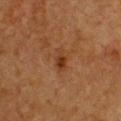Assessment:
Captured during whole-body skin photography for melanoma surveillance; the lesion was not biopsied.
Context:
A lesion tile, about 15 mm wide, cut from a 3D total-body photograph. On the front of the torso. A female patient aged around 40.